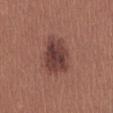Q: Automated lesion metrics?
A: a border-irregularity index near 2.5/10, a color-variation rating of about 4.5/10, and radial color variation of about 1.5; a classifier nevus-likeness of about 70/100 and lesion-presence confidence of about 100/100
Q: Lesion location?
A: the abdomen
Q: What is the imaging modality?
A: ~15 mm crop, total-body skin-cancer survey
Q: Who is the patient?
A: female, in their mid-20s
Q: How was the tile lit?
A: white-light illumination